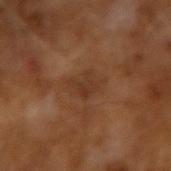Recorded during total-body skin imaging; not selected for excision or biopsy. Measured at roughly 3 mm in maximum diameter. This image is a 15 mm lesion crop taken from a total-body photograph. The subject is a male aged 63–67. This is a cross-polarized tile.